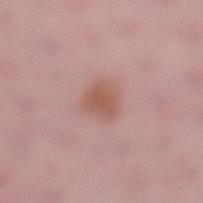biopsy status=imaged on a skin check; not biopsied